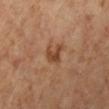| feature | finding |
|---|---|
| workup | catalogued during a skin exam; not biopsied |
| anatomic site | the left lower leg |
| illumination | cross-polarized illumination |
| subject | female, aged 63–67 |
| TBP lesion metrics | an average lesion color of about L≈43 a*≈21 b*≈32 (CIELAB), a lesion–skin lightness drop of about 9, and a normalized border contrast of about 7.5; a border-irregularity index near 4.5/10 and radial color variation of about 1 |
| acquisition | 15 mm crop, total-body photography |